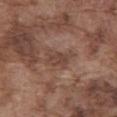The lesion was photographed on a routine skin check and not biopsied; there is no pathology result. A region of skin cropped from a whole-body photographic capture, roughly 15 mm wide. The recorded lesion diameter is about 3.5 mm. On the abdomen. An algorithmic analysis of the crop reported an average lesion color of about L≈43 a*≈18 b*≈25 (CIELAB) and a lesion-to-skin contrast of about 6 (normalized; higher = more distinct). It also reported a nevus-likeness score of about 0/100 and a detector confidence of about 95 out of 100 that the crop contains a lesion. A male subject, roughly 75 years of age.